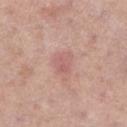Part of a total-body skin-imaging series; this lesion was reviewed on a skin check and was not flagged for biopsy.
The lesion's longest dimension is about 3 mm.
Automated tile analysis of the lesion measured a border-irregularity index near 3/10 and internal color variation of about 2.5 on a 0–10 scale. And it measured a nevus-likeness score of about 5/100 and a detector confidence of about 100 out of 100 that the crop contains a lesion.
A 15 mm close-up extracted from a 3D total-body photography capture.
A female subject in their 60s.
On the left lower leg.
The tile uses white-light illumination.